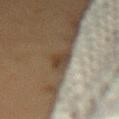Findings:
- workup: catalogued during a skin exam; not biopsied
- lesion diameter: ≈3.5 mm
- patient: female, aged around 50
- image-analysis metrics: a footprint of about 4.5 mm², a shape eccentricity near 0.85, and a symmetry-axis asymmetry near 0.35; a border-irregularity index near 3.5/10, internal color variation of about 2.5 on a 0–10 scale, and peripheral color asymmetry of about 1
- acquisition: ~15 mm tile from a whole-body skin photo
- body site: the lower back